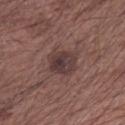{"lighting": "white-light", "image": {"source": "total-body photography crop", "field_of_view_mm": 15}, "site": "left upper arm", "lesion_size": {"long_diameter_mm_approx": 3.5}, "patient": {"sex": "male", "age_approx": 65}}